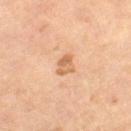No biopsy was performed on this lesion — it was imaged during a full skin examination and was not determined to be concerning.
This image is a 15 mm lesion crop taken from a total-body photograph.
A male patient aged 63 to 67.
The lesion is on the leg.